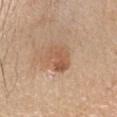{
  "biopsy_status": "not biopsied; imaged during a skin examination",
  "site": "head or neck",
  "patient": {
    "sex": "male",
    "age_approx": 40
  },
  "lighting": "white-light",
  "lesion_size": {
    "long_diameter_mm_approx": 4.0
  },
  "image": {
    "source": "total-body photography crop",
    "field_of_view_mm": 15
  }
}